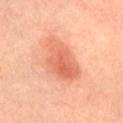The lesion was tiled from a total-body skin photograph and was not biopsied. A 15 mm close-up tile from a total-body photography series done for melanoma screening. The lesion is located on the abdomen. The subject is a female aged 28–32.